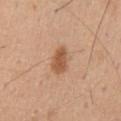* follow-up · imaged on a skin check; not biopsied
* tile lighting · white-light
* acquisition · ~15 mm tile from a whole-body skin photo
* automated metrics · a lesion color around L≈55 a*≈22 b*≈35 in CIELAB; a border-irregularity index near 2/10 and radial color variation of about 0.5
* patient · male, in their mid-40s
* lesion diameter · about 3.5 mm
* site · the mid back